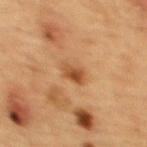Q: Is there a histopathology result?
A: imaged on a skin check; not biopsied
Q: What kind of image is this?
A: ~15 mm tile from a whole-body skin photo
Q: Patient demographics?
A: female, aged approximately 70
Q: Where on the body is the lesion?
A: the back